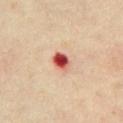Case summary:
- follow-up — imaged on a skin check; not biopsied
- image source — total-body-photography crop, ~15 mm field of view
- tile lighting — cross-polarized
- anatomic site — the chest
- size — ~2.5 mm (longest diameter)
- patient — female, in their mid-40s